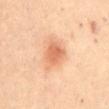  biopsy_status: not biopsied; imaged during a skin examination
  site: front of the torso
  image:
    source: total-body photography crop
    field_of_view_mm: 15
  lesion_size:
    long_diameter_mm_approx: 4.5
  lighting: cross-polarized
  patient:
    sex: female
    age_approx: 45